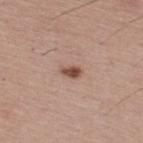No biopsy was performed on this lesion — it was imaged during a full skin examination and was not determined to be concerning. On the upper back. A close-up tile cropped from a whole-body skin photograph, about 15 mm across. The patient is a male about 40 years old.